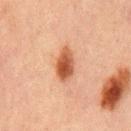| field | value |
|---|---|
| workup | no biopsy performed (imaged during a skin exam) |
| acquisition | ~15 mm crop, total-body skin-cancer survey |
| tile lighting | cross-polarized illumination |
| patient | male, aged around 65 |
| body site | the mid back |